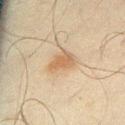Recorded during total-body skin imaging; not selected for excision or biopsy. The lesion-visualizer software estimated a mean CIELAB color near L≈51 a*≈14 b*≈31, a lesion–skin lightness drop of about 8, and a normalized border contrast of about 7. Captured under cross-polarized illumination. A region of skin cropped from a whole-body photographic capture, roughly 15 mm wide. Located on the right thigh. A male subject, aged 43–47.